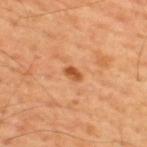– workup — catalogued during a skin exam; not biopsied